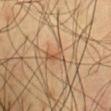follow-up=no biopsy performed (imaged during a skin exam).